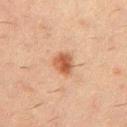| field | value |
|---|---|
| notes | no biopsy performed (imaged during a skin exam) |
| size | ~3 mm (longest diameter) |
| location | the chest |
| image source | total-body-photography crop, ~15 mm field of view |
| lighting | cross-polarized illumination |
| patient | male, aged 48 to 52 |
| image-analysis metrics | a symmetry-axis asymmetry near 0.2; border irregularity of about 1.5 on a 0–10 scale, a color-variation rating of about 4/10, and peripheral color asymmetry of about 1.5 |